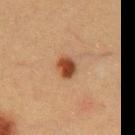Background: From the front of the torso. A male subject, aged approximately 50. A 15 mm crop from a total-body photograph taken for skin-cancer surveillance.